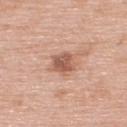Part of a total-body skin-imaging series; this lesion was reviewed on a skin check and was not flagged for biopsy. The recorded lesion diameter is about 3 mm. The lesion is on the upper back. The subject is a male aged approximately 60. A roughly 15 mm field-of-view crop from a total-body skin photograph. The total-body-photography lesion software estimated an average lesion color of about L≈57 a*≈23 b*≈29 (CIELAB), roughly 12 lightness units darker than nearby skin, and a normalized lesion–skin contrast near 7.5. The software also gave a lesion-detection confidence of about 100/100.